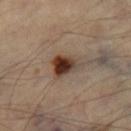Assessment:
The lesion was photographed on a routine skin check and not biopsied; there is no pathology result.
Clinical summary:
This image is a 15 mm lesion crop taken from a total-body photograph. The patient is a male about 55 years old. On the leg.